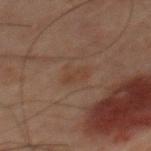Case summary:
• workup · imaged on a skin check; not biopsied
• acquisition · ~15 mm crop, total-body skin-cancer survey
• body site · the mid back
• automated lesion analysis · a lesion area of about 4.5 mm², a shape eccentricity near 0.4, and two-axis asymmetry of about 0.4; a lesion–skin lightness drop of about 4 and a normalized border contrast of about 5; a border-irregularity rating of about 4/10, a color-variation rating of about 1.5/10, and a peripheral color-asymmetry measure near 0.5
• subject · male, aged 58–62